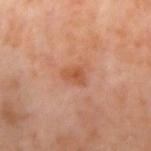Q: Was a biopsy performed?
A: catalogued during a skin exam; not biopsied
Q: What is the lesion's diameter?
A: ≈2.5 mm
Q: What kind of image is this?
A: 15 mm crop, total-body photography
Q: Where on the body is the lesion?
A: the left upper arm
Q: Automated lesion metrics?
A: an average lesion color of about L≈52 a*≈26 b*≈35 (CIELAB), about 8 CIELAB-L* units darker than the surrounding skin, and a normalized lesion–skin contrast near 6.5; a border-irregularity index near 4/10 and peripheral color asymmetry of about 0.5
Q: Patient demographics?
A: female, aged approximately 50
Q: Illumination type?
A: cross-polarized illumination